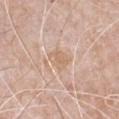Part of a total-body skin-imaging series; this lesion was reviewed on a skin check and was not flagged for biopsy. The subject is a male in their 80s. About 3 mm across. The tile uses white-light illumination. On the chest. Cropped from a total-body skin-imaging series; the visible field is about 15 mm.